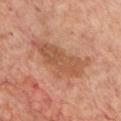  biopsy_status: not biopsied; imaged during a skin examination
  lighting: cross-polarized
  lesion_size:
    long_diameter_mm_approx: 8.0
  image:
    source: total-body photography crop
    field_of_view_mm: 15
  automated_metrics:
    eccentricity: 0.9
    cielab_L: 52
    cielab_a: 23
    cielab_b: 32
    vs_skin_darker_L: 9.0
    vs_skin_contrast_norm: 6.5
    nevus_likeness_0_100: 10
    lesion_detection_confidence_0_100: 100
  site: chest
  patient:
    sex: female
    age_approx: 45